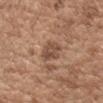Assessment:
Imaged during a routine full-body skin examination; the lesion was not biopsied and no histopathology is available.
Context:
The subject is a male roughly 70 years of age. About 3.5 mm across. A close-up tile cropped from a whole-body skin photograph, about 15 mm across. Captured under white-light illumination. Automated tile analysis of the lesion measured an eccentricity of roughly 0.7 and a shape-asymmetry score of about 0.25 (0 = symmetric). The analysis additionally found a border-irregularity index near 2.5/10. And it measured a classifier nevus-likeness of about 5/100 and a lesion-detection confidence of about 100/100. From the left upper arm.